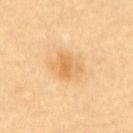Findings:
* biopsy status: no biopsy performed (imaged during a skin exam)
* site: the upper back
* patient: male, aged 83 or over
* image: ~15 mm tile from a whole-body skin photo
* diameter: ~3.5 mm (longest diameter)